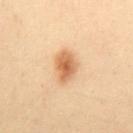• workup — imaged on a skin check; not biopsied
• subject — female, aged 38 to 42
• automated metrics — a lesion color around L≈52 a*≈19 b*≈33 in CIELAB, a lesion–skin lightness drop of about 11, and a lesion-to-skin contrast of about 8.5 (normalized; higher = more distinct)
• illumination — cross-polarized illumination
• lesion size — ~3.5 mm (longest diameter)
• acquisition — 15 mm crop, total-body photography
• anatomic site — the abdomen